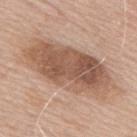Clinical impression: The lesion was tiled from a total-body skin photograph and was not biopsied. Clinical summary: The lesion's longest dimension is about 11 mm. Captured under white-light illumination. The lesion is on the upper back. Automated image analysis of the tile measured an area of roughly 43 mm². It also reported an average lesion color of about L≈56 a*≈19 b*≈29 (CIELAB) and roughly 13 lightness units darker than nearby skin. The software also gave a classifier nevus-likeness of about 30/100 and a detector confidence of about 100 out of 100 that the crop contains a lesion. This image is a 15 mm lesion crop taken from a total-body photograph. A male subject approximately 65 years of age.